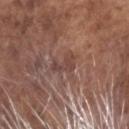Impression:
Part of a total-body skin-imaging series; this lesion was reviewed on a skin check and was not flagged for biopsy.